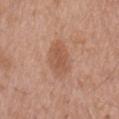Q: Was a biopsy performed?
A: imaged on a skin check; not biopsied
Q: How large is the lesion?
A: about 5 mm
Q: Patient demographics?
A: male, in their mid- to late 70s
Q: What is the imaging modality?
A: total-body-photography crop, ~15 mm field of view
Q: Where on the body is the lesion?
A: the mid back